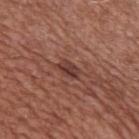biopsy status: imaged on a skin check; not biopsied
imaging modality: ~15 mm tile from a whole-body skin photo
anatomic site: the arm
illumination: white-light
patient: male, aged 53 to 57